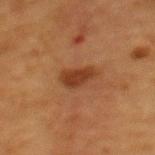notes: catalogued during a skin exam; not biopsied | image source: total-body-photography crop, ~15 mm field of view | anatomic site: the mid back | image-analysis metrics: a footprint of about 6 mm² and a shape eccentricity near 0.8; an average lesion color of about L≈32 a*≈21 b*≈30 (CIELAB), a lesion–skin lightness drop of about 9, and a lesion-to-skin contrast of about 8.5 (normalized; higher = more distinct); a border-irregularity rating of about 3/10, a color-variation rating of about 2/10, and radial color variation of about 0.5; a classifier nevus-likeness of about 95/100 and lesion-presence confidence of about 100/100 | lesion diameter: ~3.5 mm (longest diameter) | subject: female, aged approximately 50 | tile lighting: cross-polarized.